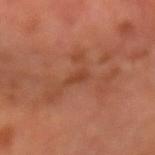The lesion was tiled from a total-body skin photograph and was not biopsied. A male patient in their mid-60s. About 2.5 mm across. A 15 mm close-up extracted from a 3D total-body photography capture. The total-body-photography lesion software estimated a lesion area of about 2.5 mm² and an eccentricity of roughly 0.85. And it measured a lesion color around L≈42 a*≈26 b*≈32 in CIELAB, a lesion–skin lightness drop of about 6, and a normalized lesion–skin contrast near 5.5. The analysis additionally found a detector confidence of about 95 out of 100 that the crop contains a lesion.